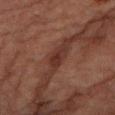Impression:
The lesion was photographed on a routine skin check and not biopsied; there is no pathology result.
Clinical summary:
A roughly 15 mm field-of-view crop from a total-body skin photograph. The lesion is located on the left lower leg. Imaged with cross-polarized lighting. The recorded lesion diameter is about 3.5 mm. Automated tile analysis of the lesion measured a footprint of about 4 mm², an eccentricity of roughly 0.9, and a symmetry-axis asymmetry near 0.35. The software also gave a lesion color around L≈27 a*≈20 b*≈22 in CIELAB and about 8 CIELAB-L* units darker than the surrounding skin. The subject is a male roughly 85 years of age or older.